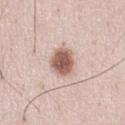Context: The lesion's longest dimension is about 4 mm. The lesion is located on the front of the torso. A 15 mm close-up extracted from a 3D total-body photography capture. A male patient in their mid-30s. This is a white-light tile.